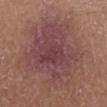Q: Is there a histopathology result?
A: total-body-photography surveillance lesion; no biopsy
Q: How was this image acquired?
A: total-body-photography crop, ~15 mm field of view
Q: Automated lesion metrics?
A: a lesion color around L≈45 a*≈21 b*≈20 in CIELAB, about 8 CIELAB-L* units darker than the surrounding skin, and a normalized border contrast of about 7.5; a border-irregularity rating of about 4.5/10 and a color-variation rating of about 5/10; an automated nevus-likeness rating near 10 out of 100 and lesion-presence confidence of about 100/100
Q: How large is the lesion?
A: ~10.5 mm (longest diameter)
Q: Patient demographics?
A: male, approximately 65 years of age
Q: Where on the body is the lesion?
A: the right lower leg
Q: Illumination type?
A: white-light